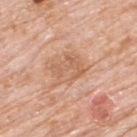Notes:
• biopsy status — catalogued during a skin exam; not biopsied
• patient — male, aged 78–82
• illumination — white-light
• body site — the upper back
• image source — 15 mm crop, total-body photography
• lesion diameter — about 3.5 mm
• image-analysis metrics — an area of roughly 9 mm², a shape eccentricity near 0.55, and two-axis asymmetry of about 0.2; an automated nevus-likeness rating near 0 out of 100